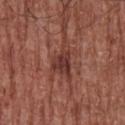Captured during whole-body skin photography for melanoma surveillance; the lesion was not biopsied. A male patient in their mid-70s. Located on the chest. A roughly 15 mm field-of-view crop from a total-body skin photograph.